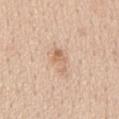  biopsy_status: not biopsied; imaged during a skin examination
  patient:
    sex: male
    age_approx: 60
  image:
    source: total-body photography crop
    field_of_view_mm: 15
  automated_metrics:
    area_mm2_approx: 4.5
    eccentricity: 0.9
    shape_asymmetry: 0.3
    border_irregularity_0_10: 4.5
    color_variation_0_10: 2.5
    peripheral_color_asymmetry: 0.5
    nevus_likeness_0_100: 5
  site: upper back
  lesion_size:
    long_diameter_mm_approx: 3.5
  lighting: white-light